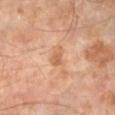workup: catalogued during a skin exam; not biopsied
image: ~15 mm tile from a whole-body skin photo
subject: male, aged 58–62
automated lesion analysis: a border-irregularity rating of about 4/10, internal color variation of about 1.5 on a 0–10 scale, and peripheral color asymmetry of about 0.5
lesion diameter: ~2.5 mm (longest diameter)
body site: the left lower leg